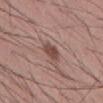{"biopsy_status": "not biopsied; imaged during a skin examination", "lesion_size": {"long_diameter_mm_approx": 3.0}, "patient": {"sex": "male", "age_approx": 35}, "site": "mid back", "automated_metrics": {"border_irregularity_0_10": 2.0, "color_variation_0_10": 1.5, "peripheral_color_asymmetry": 0.5}, "image": {"source": "total-body photography crop", "field_of_view_mm": 15}}